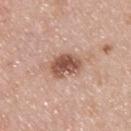Clinical impression:
Recorded during total-body skin imaging; not selected for excision or biopsy.
Background:
About 4 mm across. A male subject roughly 45 years of age. A region of skin cropped from a whole-body photographic capture, roughly 15 mm wide. Located on the upper back.